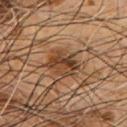Findings:
* image source — ~15 mm crop, total-body skin-cancer survey
* patient — male, aged around 55
* body site — the chest
* lesion diameter — ~4 mm (longest diameter)
* tile lighting — cross-polarized illumination